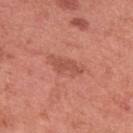{"biopsy_status": "not biopsied; imaged during a skin examination", "site": "arm", "patient": {"sex": "female", "age_approx": 50}, "image": {"source": "total-body photography crop", "field_of_view_mm": 15}}